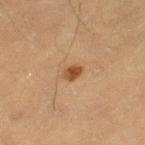No biopsy was performed on this lesion — it was imaged during a full skin examination and was not determined to be concerning. A 15 mm crop from a total-body photograph taken for skin-cancer surveillance. The patient is a male aged around 60. This is a cross-polarized tile. Longest diameter approximately 2.5 mm. From the left thigh.